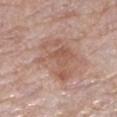The lesion was tiled from a total-body skin photograph and was not biopsied.